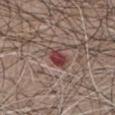follow-up: catalogued during a skin exam; not biopsied